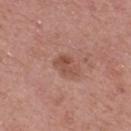– biopsy status — catalogued during a skin exam; not biopsied
– lighting — white-light illumination
– automated lesion analysis — a mean CIELAB color near L≈50 a*≈23 b*≈27, about 9 CIELAB-L* units darker than the surrounding skin, and a normalized lesion–skin contrast near 6.5; a border-irregularity rating of about 4.5/10, a color-variation rating of about 2.5/10, and radial color variation of about 1; a nevus-likeness score of about 10/100 and a lesion-detection confidence of about 100/100
– image — 15 mm crop, total-body photography
– lesion diameter — about 3 mm
– subject — male, aged around 65
– location — the back